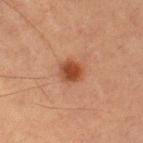Captured during whole-body skin photography for melanoma surveillance; the lesion was not biopsied. This image is a 15 mm lesion crop taken from a total-body photograph. Measured at roughly 2.5 mm in maximum diameter. The lesion is located on the left thigh. A male patient in their mid-80s or older.